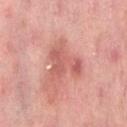The lesion was photographed on a routine skin check and not biopsied; there is no pathology result. Located on the abdomen. A female subject aged approximately 50. A close-up tile cropped from a whole-body skin photograph, about 15 mm across.